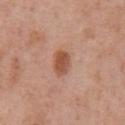Impression: Recorded during total-body skin imaging; not selected for excision or biopsy. Clinical summary: Approximately 3 mm at its widest. The lesion is located on the chest. The tile uses white-light illumination. A male patient in their 70s. This image is a 15 mm lesion crop taken from a total-body photograph. The total-body-photography lesion software estimated a border-irregularity rating of about 2/10, internal color variation of about 3.5 on a 0–10 scale, and peripheral color asymmetry of about 1. It also reported an automated nevus-likeness rating near 100 out of 100 and a lesion-detection confidence of about 100/100.